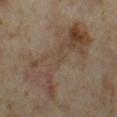{
  "biopsy_status": "not biopsied; imaged during a skin examination",
  "lighting": "cross-polarized",
  "site": "left thigh",
  "automated_metrics": {
    "area_mm2_approx": 32.0,
    "eccentricity": 0.85,
    "shape_asymmetry": 0.4,
    "cielab_L": 41,
    "cielab_a": 13,
    "cielab_b": 25,
    "vs_skin_contrast_norm": 6.0,
    "color_variation_0_10": 7.5,
    "nevus_likeness_0_100": 0,
    "lesion_detection_confidence_0_100": 65
  },
  "image": {
    "source": "total-body photography crop",
    "field_of_view_mm": 15
  },
  "lesion_size": {
    "long_diameter_mm_approx": 9.0
  },
  "patient": {
    "sex": "female",
    "age_approx": 35
  }
}